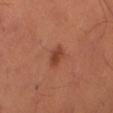Clinical impression:
Recorded during total-body skin imaging; not selected for excision or biopsy.
Image and clinical context:
This is a cross-polarized tile. A 15 mm crop from a total-body photograph taken for skin-cancer surveillance. The subject is a male aged around 55. The lesion is on the right thigh.